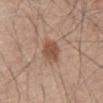{
  "biopsy_status": "not biopsied; imaged during a skin examination",
  "lesion_size": {
    "long_diameter_mm_approx": 3.5
  },
  "site": "abdomen",
  "image": {
    "source": "total-body photography crop",
    "field_of_view_mm": 15
  },
  "patient": {
    "sex": "male",
    "age_approx": 80
  },
  "lighting": "white-light"
}